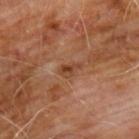Captured during whole-body skin photography for melanoma surveillance; the lesion was not biopsied. Captured under cross-polarized illumination. A male subject in their 60s. On the front of the torso. The lesion-visualizer software estimated border irregularity of about 3.5 on a 0–10 scale, internal color variation of about 4 on a 0–10 scale, and radial color variation of about 1.5. The lesion's longest dimension is about 2.5 mm. This image is a 15 mm lesion crop taken from a total-body photograph.